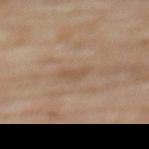Recorded during total-body skin imaging; not selected for excision or biopsy.
A 15 mm crop from a total-body photograph taken for skin-cancer surveillance.
A female patient, aged approximately 75.
About 2.5 mm across.
From the front of the torso.
The tile uses cross-polarized illumination.
The total-body-photography lesion software estimated a mean CIELAB color near L≈52 a*≈16 b*≈30, roughly 6 lightness units darker than nearby skin, and a normalized border contrast of about 5. It also reported a nevus-likeness score of about 0/100 and a detector confidence of about 95 out of 100 that the crop contains a lesion.